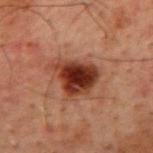Captured under cross-polarized illumination. A male patient, roughly 60 years of age. The lesion is located on the mid back. Cropped from a total-body skin-imaging series; the visible field is about 15 mm. About 5.5 mm across.